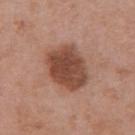| field | value |
|---|---|
| site | the abdomen |
| acquisition | ~15 mm crop, total-body skin-cancer survey |
| image-analysis metrics | a normalized lesion–skin contrast near 10; a classifier nevus-likeness of about 65/100 and a detector confidence of about 100 out of 100 that the crop contains a lesion |
| subject | male, about 70 years old |
| size | ~5 mm (longest diameter) |